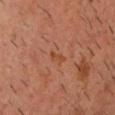No biopsy was performed on this lesion — it was imaged during a full skin examination and was not determined to be concerning.
This image is a 15 mm lesion crop taken from a total-body photograph.
The recorded lesion diameter is about 2.5 mm.
The tile uses cross-polarized illumination.
On the head or neck.
The subject is a male aged 48–52.